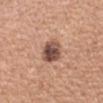<lesion>
  <biopsy_status>not biopsied; imaged during a skin examination</biopsy_status>
  <lesion_size>
    <long_diameter_mm_approx>3.0</long_diameter_mm_approx>
  </lesion_size>
  <lighting>white-light</lighting>
  <image>
    <source>total-body photography crop</source>
    <field_of_view_mm>15</field_of_view_mm>
  </image>
  <patient>
    <sex>female</sex>
    <age_approx>35</age_approx>
  </patient>
  <automated_metrics>
    <area_mm2_approx>8.0</area_mm2_approx>
    <cielab_L>50</cielab_L>
    <cielab_a>21</cielab_a>
    <cielab_b>25</cielab_b>
    <vs_skin_contrast_norm>11.5</vs_skin_contrast_norm>
  </automated_metrics>
  <site>left upper arm</site>
</lesion>